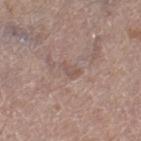follow-up: imaged on a skin check; not biopsied
automated lesion analysis: a shape eccentricity near 0.9 and a symmetry-axis asymmetry near 0.35; a border-irregularity index near 3.5/10, a within-lesion color-variation index near 0.5/10, and a peripheral color-asymmetry measure near 0
lighting: white-light illumination
image source: ~15 mm crop, total-body skin-cancer survey
subject: male, about 65 years old
size: about 2.5 mm
body site: the left thigh